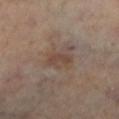Q: How was the tile lit?
A: cross-polarized illumination
Q: What kind of image is this?
A: total-body-photography crop, ~15 mm field of view
Q: Who is the patient?
A: female, approximately 60 years of age
Q: Automated lesion metrics?
A: a lesion-to-skin contrast of about 6 (normalized; higher = more distinct); a border-irregularity rating of about 3.5/10 and a color-variation rating of about 1.5/10
Q: What is the anatomic site?
A: the leg
Q: Lesion size?
A: about 3 mm